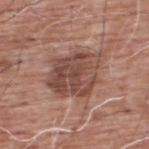Q: Was this lesion biopsied?
A: total-body-photography surveillance lesion; no biopsy
Q: What is the anatomic site?
A: the upper back
Q: What is the lesion's diameter?
A: ≈5.5 mm
Q: Automated lesion metrics?
A: border irregularity of about 3 on a 0–10 scale, a color-variation rating of about 5.5/10, and peripheral color asymmetry of about 2; a classifier nevus-likeness of about 10/100 and a detector confidence of about 100 out of 100 that the crop contains a lesion
Q: How was the tile lit?
A: white-light
Q: Patient demographics?
A: male, in their 60s
Q: What is the imaging modality?
A: total-body-photography crop, ~15 mm field of view